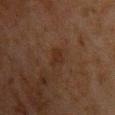Impression:
Part of a total-body skin-imaging series; this lesion was reviewed on a skin check and was not flagged for biopsy.
Background:
A male patient, aged 58–62. Located on the front of the torso. A 15 mm close-up tile from a total-body photography series done for melanoma screening. This is a cross-polarized tile.